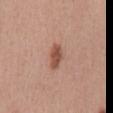follow-up — catalogued during a skin exam; not biopsied
patient — male, roughly 55 years of age
imaging modality — ~15 mm crop, total-body skin-cancer survey
site — the chest